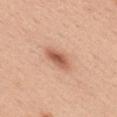A female subject aged 38 to 42. Imaged with white-light lighting. An algorithmic analysis of the crop reported an area of roughly 6 mm², an eccentricity of roughly 0.85, and a shape-asymmetry score of about 0.2 (0 = symmetric). The analysis additionally found a lesion-to-skin contrast of about 8 (normalized; higher = more distinct). Measured at roughly 3.5 mm in maximum diameter. The lesion is on the back. A close-up tile cropped from a whole-body skin photograph, about 15 mm across.